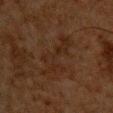{
  "biopsy_status": "not biopsied; imaged during a skin examination",
  "site": "chest",
  "image": {
    "source": "total-body photography crop",
    "field_of_view_mm": 15
  },
  "patient": {
    "sex": "male",
    "age_approx": 65
  }
}